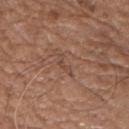Impression: Recorded during total-body skin imaging; not selected for excision or biopsy. Background: A male patient, aged approximately 75. This is a white-light tile. Located on the left upper arm. This image is a 15 mm lesion crop taken from a total-body photograph. The lesion's longest dimension is about 3 mm. An algorithmic analysis of the crop reported an eccentricity of roughly 0.9 and a shape-asymmetry score of about 0.65 (0 = symmetric). The software also gave a classifier nevus-likeness of about 0/100 and lesion-presence confidence of about 60/100.